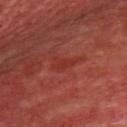Clinical impression:
Recorded during total-body skin imaging; not selected for excision or biopsy.
Image and clinical context:
A male subject aged 58–62. Located on the chest. About 3 mm across. A 15 mm crop from a total-body photograph taken for skin-cancer surveillance. The tile uses cross-polarized illumination.